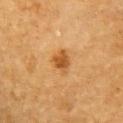follow-up — imaged on a skin check; not biopsied
TBP lesion metrics — a mean CIELAB color near L≈47 a*≈22 b*≈41, a lesion–skin lightness drop of about 11, and a normalized lesion–skin contrast near 8.5; a border-irregularity index near 2.5/10, a within-lesion color-variation index near 2/10, and radial color variation of about 0.5
image source — ~15 mm crop, total-body skin-cancer survey
location — the right upper arm
subject — male, in their mid- to late 80s or older
tile lighting — cross-polarized illumination
diameter — about 2.5 mm